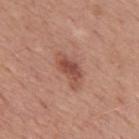notes — no biopsy performed (imaged during a skin exam) | acquisition — 15 mm crop, total-body photography | TBP lesion metrics — an eccentricity of roughly 0.9 and two-axis asymmetry of about 0.4; a mean CIELAB color near L≈50 a*≈25 b*≈28 and roughly 10 lightness units darker than nearby skin; internal color variation of about 3.5 on a 0–10 scale; a classifier nevus-likeness of about 75/100 and a detector confidence of about 100 out of 100 that the crop contains a lesion | body site — the mid back | subject — male, aged approximately 50 | diameter — ~4.5 mm (longest diameter).